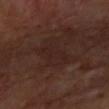<tbp_lesion>
  <biopsy_status>not biopsied; imaged during a skin examination</biopsy_status>
  <lesion_size>
    <long_diameter_mm_approx>5.0</long_diameter_mm_approx>
  </lesion_size>
  <site>left forearm</site>
  <patient>
    <sex>male</sex>
    <age_approx>65</age_approx>
  </patient>
  <automated_metrics>
    <area_mm2_approx>9.0</area_mm2_approx>
    <eccentricity>0.9</eccentricity>
    <shape_asymmetry>0.25</shape_asymmetry>
    <cielab_L>22</cielab_L>
    <cielab_a>17</cielab_a>
    <cielab_b>19</cielab_b>
    <vs_skin_darker_L>4.0</vs_skin_darker_L>
    <vs_skin_contrast_norm>4.5</vs_skin_contrast_norm>
  </automated_metrics>
  <image>
    <source>total-body photography crop</source>
    <field_of_view_mm>15</field_of_view_mm>
  </image>
</tbp_lesion>